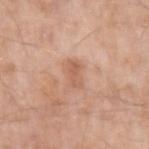biopsy status — imaged on a skin check; not biopsied | subject — male, in their mid-50s | diameter — ≈3 mm | acquisition — total-body-photography crop, ~15 mm field of view | illumination — white-light | anatomic site — the left upper arm.